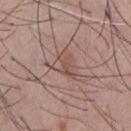Q: Is there a histopathology result?
A: catalogued during a skin exam; not biopsied
Q: What kind of image is this?
A: ~15 mm crop, total-body skin-cancer survey
Q: What lighting was used for the tile?
A: white-light
Q: How large is the lesion?
A: about 3.5 mm
Q: Who is the patient?
A: male, in their mid- to late 50s
Q: Lesion location?
A: the chest